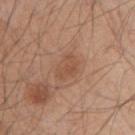Recorded during total-body skin imaging; not selected for excision or biopsy. Longest diameter approximately 3 mm. Cropped from a whole-body photographic skin survey; the tile spans about 15 mm. A male subject, in their mid-40s. On the right upper arm.